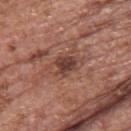The lesion is on the upper back. Automated tile analysis of the lesion measured an automated nevus-likeness rating near 15 out of 100. The tile uses white-light illumination. Approximately 2.5 mm at its widest. A region of skin cropped from a whole-body photographic capture, roughly 15 mm wide. The patient is a female in their mid- to late 60s.